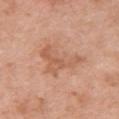Captured during whole-body skin photography for melanoma surveillance; the lesion was not biopsied.
An algorithmic analysis of the crop reported an average lesion color of about L≈59 a*≈23 b*≈33 (CIELAB) and a normalized border contrast of about 5.5. The software also gave an automated nevus-likeness rating near 0 out of 100 and a lesion-detection confidence of about 100/100.
On the chest.
Measured at roughly 6.5 mm in maximum diameter.
A roughly 15 mm field-of-view crop from a total-body skin photograph.
Captured under white-light illumination.
A female patient, about 50 years old.